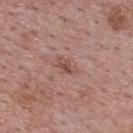Clinical impression: This lesion was catalogued during total-body skin photography and was not selected for biopsy. Clinical summary: An algorithmic analysis of the crop reported a border-irregularity index near 3.5/10 and radial color variation of about 0. Located on the upper back. A region of skin cropped from a whole-body photographic capture, roughly 15 mm wide. A male patient, aged approximately 70. Imaged with white-light lighting. About 3 mm across.